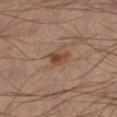Impression: Part of a total-body skin-imaging series; this lesion was reviewed on a skin check and was not flagged for biopsy. Acquisition and patient details: Automated tile analysis of the lesion measured a footprint of about 3.5 mm² and a shape-asymmetry score of about 0.25 (0 = symmetric). And it measured an average lesion color of about L≈41 a*≈18 b*≈28 (CIELAB) and about 9 CIELAB-L* units darker than the surrounding skin. The lesion's longest dimension is about 2.5 mm. Cropped from a total-body skin-imaging series; the visible field is about 15 mm. Imaged with cross-polarized lighting. The lesion is on the left lower leg. A male subject, aged approximately 45.